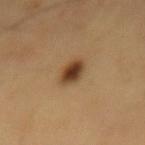<record>
<site>mid back</site>
<lesion_size>
  <long_diameter_mm_approx>3.5</long_diameter_mm_approx>
</lesion_size>
<lighting>cross-polarized</lighting>
<patient>
  <sex>male</sex>
  <age_approx>60</age_approx>
</patient>
<image>
  <source>total-body photography crop</source>
  <field_of_view_mm>15</field_of_view_mm>
</image>
<automated_metrics>
  <area_mm2_approx>6.0</area_mm2_approx>
  <shape_asymmetry>0.15</shape_asymmetry>
  <border_irregularity_0_10>1.5</border_irregularity_0_10>
  <color_variation_0_10>6.0</color_variation_0_10>
  <peripheral_color_asymmetry>1.5</peripheral_color_asymmetry>
  <nevus_likeness_0_100>100</nevus_likeness_0_100>
  <lesion_detection_confidence_0_100>100</lesion_detection_confidence_0_100>
</automated_metrics>
</record>